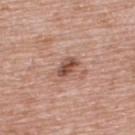Q: Was this lesion biopsied?
A: total-body-photography surveillance lesion; no biopsy
Q: Who is the patient?
A: male, aged 68–72
Q: How was the tile lit?
A: white-light
Q: Lesion location?
A: the upper back
Q: How was this image acquired?
A: total-body-photography crop, ~15 mm field of view
Q: How large is the lesion?
A: ≈2.5 mm
Q: Automated lesion metrics?
A: a lesion area of about 3.5 mm², an outline eccentricity of about 0.8 (0 = round, 1 = elongated), and two-axis asymmetry of about 0.15; a nevus-likeness score of about 20/100